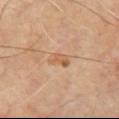Recorded during total-body skin imaging; not selected for excision or biopsy. A roughly 15 mm field-of-view crop from a total-body skin photograph. The subject is a male aged around 70. The lesion is on the front of the torso.